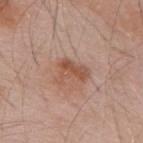<lesion>
  <biopsy_status>not biopsied; imaged during a skin examination</biopsy_status>
  <lesion_size>
    <long_diameter_mm_approx>3.5</long_diameter_mm_approx>
  </lesion_size>
  <site>back</site>
  <patient>
    <sex>male</sex>
    <age_approx>55</age_approx>
  </patient>
  <image>
    <source>total-body photography crop</source>
    <field_of_view_mm>15</field_of_view_mm>
  </image>
  <lighting>white-light</lighting>
</lesion>